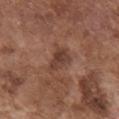Imaged during a routine full-body skin examination; the lesion was not biopsied and no histopathology is available. The subject is a male in their mid-70s. Located on the chest. Measured at roughly 3 mm in maximum diameter. This is a white-light tile. Cropped from a total-body skin-imaging series; the visible field is about 15 mm.